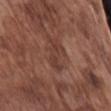Q: Is there a histopathology result?
A: imaged on a skin check; not biopsied
Q: How was this image acquired?
A: 15 mm crop, total-body photography
Q: Where on the body is the lesion?
A: the arm
Q: Who is the patient?
A: male, aged 73–77
Q: Illumination type?
A: white-light illumination
Q: Lesion size?
A: about 4 mm